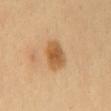- location: the lower back
- lesion diameter: about 4 mm
- acquisition: ~15 mm tile from a whole-body skin photo
- patient: female, aged approximately 50
- lighting: cross-polarized illumination